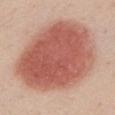Q: Was a biopsy performed?
A: total-body-photography surveillance lesion; no biopsy
Q: What kind of image is this?
A: 15 mm crop, total-body photography
Q: What is the lesion's diameter?
A: about 10.5 mm
Q: Who is the patient?
A: female, aged approximately 50
Q: Where on the body is the lesion?
A: the chest
Q: What lighting was used for the tile?
A: white-light